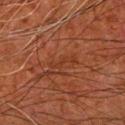Q: Was a biopsy performed?
A: catalogued during a skin exam; not biopsied
Q: Patient demographics?
A: male, aged approximately 80
Q: Illumination type?
A: cross-polarized
Q: What is the imaging modality?
A: 15 mm crop, total-body photography
Q: What is the anatomic site?
A: the arm
Q: How large is the lesion?
A: ~3.5 mm (longest diameter)